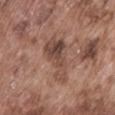| key | value |
|---|---|
| biopsy status | total-body-photography surveillance lesion; no biopsy |
| imaging modality | 15 mm crop, total-body photography |
| patient | male, aged 73–77 |
| lighting | white-light illumination |
| diameter | ~6 mm (longest diameter) |
| body site | the abdomen |